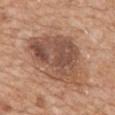The patient is a female about 70 years old.
The recorded lesion diameter is about 8.5 mm.
This image is a 15 mm lesion crop taken from a total-body photograph.
The lesion is located on the back.
This is a white-light tile.